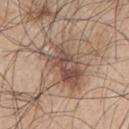follow-up: no biopsy performed (imaged during a skin exam)
patient: male, roughly 70 years of age
anatomic site: the arm
image: total-body-photography crop, ~15 mm field of view
image-analysis metrics: an area of roughly 18 mm² and a shape eccentricity near 0.85; a mean CIELAB color near L≈51 a*≈17 b*≈26, about 10 CIELAB-L* units darker than the surrounding skin, and a lesion-to-skin contrast of about 7.5 (normalized; higher = more distinct)
lesion size: about 6.5 mm
tile lighting: white-light illumination